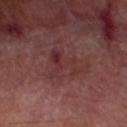The lesion was photographed on a routine skin check and not biopsied; there is no pathology result.
On the left lower leg.
A 15 mm crop from a total-body photograph taken for skin-cancer surveillance.
The subject is a male aged 68 to 72.
Automated tile analysis of the lesion measured a footprint of about 9.5 mm² and a symmetry-axis asymmetry near 0.65.
Approximately 5 mm at its widest.